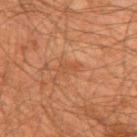This lesion was catalogued during total-body skin photography and was not selected for biopsy. From the upper back. A region of skin cropped from a whole-body photographic capture, roughly 15 mm wide. A male patient in their 60s. Captured under cross-polarized illumination. About 2.5 mm across.